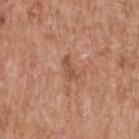The lesion was photographed on a routine skin check and not biopsied; there is no pathology result.
Cropped from a total-body skin-imaging series; the visible field is about 15 mm.
Captured under white-light illumination.
From the upper back.
A male patient in their 60s.